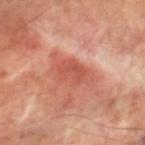<case>
<biopsy_status>not biopsied; imaged during a skin examination</biopsy_status>
<image>
  <source>total-body photography crop</source>
  <field_of_view_mm>15</field_of_view_mm>
</image>
<patient>
  <sex>male</sex>
  <age_approx>70</age_approx>
</patient>
<automated_metrics>
  <area_mm2_approx>7.5</area_mm2_approx>
  <eccentricity>0.8</eccentricity>
  <shape_asymmetry>0.2</shape_asymmetry>
  <cielab_L>53</cielab_L>
  <cielab_a>31</cielab_a>
  <cielab_b>32</cielab_b>
  <vs_skin_darker_L>9.0</vs_skin_darker_L>
  <color_variation_0_10>2.5</color_variation_0_10>
  <peripheral_color_asymmetry>1.0</peripheral_color_asymmetry>
  <nevus_likeness_0_100>0</nevus_likeness_0_100>
  <lesion_detection_confidence_0_100>70</lesion_detection_confidence_0_100>
</automated_metrics>
<lighting>cross-polarized</lighting>
<site>left thigh</site>
<lesion_size>
  <long_diameter_mm_approx>4.0</long_diameter_mm_approx>
</lesion_size>
</case>